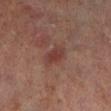Recorded during total-body skin imaging; not selected for excision or biopsy.
A 15 mm close-up tile from a total-body photography series done for melanoma screening.
The lesion is located on the left lower leg.
A male subject aged approximately 70.